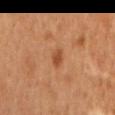The lesion was photographed on a routine skin check and not biopsied; there is no pathology result.
A male patient aged 58 to 62.
An algorithmic analysis of the crop reported a lesion area of about 3 mm² and two-axis asymmetry of about 0.3. It also reported a border-irregularity index near 2.5/10, a color-variation rating of about 1.5/10, and radial color variation of about 0.5. It also reported a nevus-likeness score of about 70/100 and lesion-presence confidence of about 100/100.
A 15 mm crop from a total-body photograph taken for skin-cancer surveillance.
From the chest.
This is a cross-polarized tile.
Longest diameter approximately 2.5 mm.